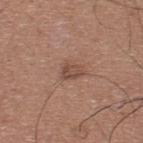size: ≈2.5 mm | image source: ~15 mm tile from a whole-body skin photo | TBP lesion metrics: a footprint of about 4 mm², a shape eccentricity near 0.7, and a symmetry-axis asymmetry near 0.35; an average lesion color of about L≈47 a*≈20 b*≈26 (CIELAB), a lesion–skin lightness drop of about 9, and a normalized lesion–skin contrast near 6.5; a border-irregularity index near 3/10 and internal color variation of about 3 on a 0–10 scale; a nevus-likeness score of about 30/100 | tile lighting: white-light illumination | anatomic site: the upper back | subject: male, aged around 35.